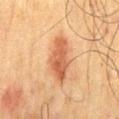Notes:
– follow-up: total-body-photography surveillance lesion; no biopsy
– imaging modality: 15 mm crop, total-body photography
– patient: male, roughly 70 years of age
– location: the mid back